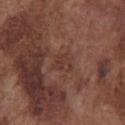notes = total-body-photography surveillance lesion; no biopsy
image = ~15 mm tile from a whole-body skin photo
location = the chest
automated lesion analysis = a lesion area of about 4.5 mm² and an eccentricity of roughly 0.55; an average lesion color of about L≈37 a*≈21 b*≈25 (CIELAB), about 5 CIELAB-L* units darker than the surrounding skin, and a normalized border contrast of about 5; a border-irregularity rating of about 5/10
illumination = white-light illumination
lesion diameter = ~2.5 mm (longest diameter)
patient = male, roughly 75 years of age